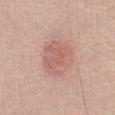Part of a total-body skin-imaging series; this lesion was reviewed on a skin check and was not flagged for biopsy. The lesion is located on the chest. Cropped from a total-body skin-imaging series; the visible field is about 15 mm. A male subject, roughly 40 years of age.